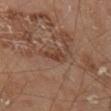Impression: Imaged during a routine full-body skin examination; the lesion was not biopsied and no histopathology is available. Clinical summary: A 15 mm close-up extracted from a 3D total-body photography capture. The tile uses cross-polarized illumination. Located on the right thigh. A male subject about 70 years old.